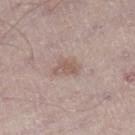notes: no biopsy performed (imaged during a skin exam)
lesion size: about 3 mm
imaging modality: total-body-photography crop, ~15 mm field of view
illumination: white-light illumination
image-analysis metrics: a lesion color around L≈56 a*≈16 b*≈24 in CIELAB, a lesion–skin lightness drop of about 8, and a lesion-to-skin contrast of about 6.5 (normalized; higher = more distinct); a within-lesion color-variation index near 1/10 and radial color variation of about 0.5
body site: the right lower leg
patient: male, aged 68–72